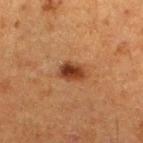notes — total-body-photography surveillance lesion; no biopsy
patient — female, aged 48–52
automated lesion analysis — lesion-presence confidence of about 100/100
imaging modality — total-body-photography crop, ~15 mm field of view
lesion diameter — ~3 mm (longest diameter)
illumination — cross-polarized illumination
anatomic site — the left lower leg